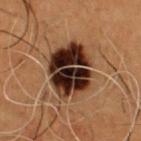Imaged during a routine full-body skin examination; the lesion was not biopsied and no histopathology is available. The lesion is located on the front of the torso. A male patient aged around 50. A region of skin cropped from a whole-body photographic capture, roughly 15 mm wide. About 6 mm across.